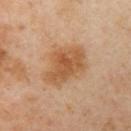A lesion tile, about 15 mm wide, cut from a 3D total-body photograph.
The tile uses cross-polarized illumination.
From the arm.
A female patient, roughly 40 years of age.
An algorithmic analysis of the crop reported a lesion color around L≈57 a*≈21 b*≈37 in CIELAB and a normalized lesion–skin contrast near 7.5.
Approximately 6 mm at its widest.